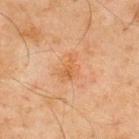{
  "biopsy_status": "not biopsied; imaged during a skin examination",
  "image": {
    "source": "total-body photography crop",
    "field_of_view_mm": 15
  },
  "site": "back",
  "patient": {
    "sex": "male",
    "age_approx": 45
  }
}